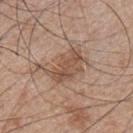Notes:
– biopsy status — no biopsy performed (imaged during a skin exam)
– imaging modality — ~15 mm crop, total-body skin-cancer survey
– anatomic site — the chest
– subject — male, about 50 years old
– diameter — about 5.5 mm